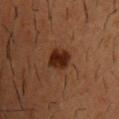{
  "biopsy_status": "not biopsied; imaged during a skin examination",
  "patient": {
    "sex": "male",
    "age_approx": 55
  },
  "site": "front of the torso",
  "image": {
    "source": "total-body photography crop",
    "field_of_view_mm": 15
  }
}